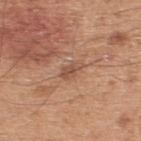workup = catalogued during a skin exam; not biopsied | tile lighting = white-light | site = the upper back | TBP lesion metrics = an average lesion color of about L≈51 a*≈21 b*≈30 (CIELAB), a lesion–skin lightness drop of about 9, and a normalized border contrast of about 6.5; an automated nevus-likeness rating near 0 out of 100 and a lesion-detection confidence of about 100/100 | lesion diameter = ~3 mm (longest diameter) | image = ~15 mm crop, total-body skin-cancer survey | subject = male, in their mid-40s.